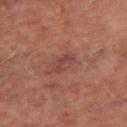workup: total-body-photography surveillance lesion; no biopsy | illumination: cross-polarized | automated lesion analysis: a shape eccentricity near 0.9 and a shape-asymmetry score of about 0.25 (0 = symmetric); a mean CIELAB color near L≈34 a*≈21 b*≈20, roughly 6 lightness units darker than nearby skin, and a normalized lesion–skin contrast near 5.5; a color-variation rating of about 2.5/10 and peripheral color asymmetry of about 1; an automated nevus-likeness rating near 0 out of 100 and lesion-presence confidence of about 90/100 | location: the leg | imaging modality: total-body-photography crop, ~15 mm field of view | size: about 3.5 mm | patient: male, aged approximately 70.